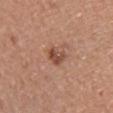  biopsy_status: not biopsied; imaged during a skin examination
  image:
    source: total-body photography crop
    field_of_view_mm: 15
  site: front of the torso
  automated_metrics:
    border_irregularity_0_10: 2.5
    color_variation_0_10: 3.0
    peripheral_color_asymmetry: 1.0
  patient:
    sex: male
    age_approx: 40
  lesion_size:
    long_diameter_mm_approx: 3.0
  lighting: white-light